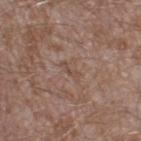Q: Was a biopsy performed?
A: no biopsy performed (imaged during a skin exam)
Q: Where on the body is the lesion?
A: the leg
Q: Who is the patient?
A: male, about 45 years old
Q: What is the imaging modality?
A: 15 mm crop, total-body photography